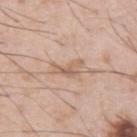automated_metrics:
  area_mm2_approx: 4.5
  eccentricity: 0.85
  shape_asymmetry: 0.6
  vs_skin_darker_L: 9.0
  nevus_likeness_0_100: 0
image:
  source: total-body photography crop
  field_of_view_mm: 15
lighting: white-light
lesion_size:
  long_diameter_mm_approx: 3.5
patient:
  sex: male
  age_approx: 55